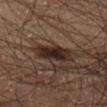workup=catalogued during a skin exam; not biopsied
illumination=cross-polarized illumination
site=the left thigh
image source=total-body-photography crop, ~15 mm field of view
size=~4.5 mm (longest diameter)
subject=male, aged 53 to 57
TBP lesion metrics=a lesion color around L≈23 a*≈14 b*≈19 in CIELAB and a normalized border contrast of about 12.5; a classifier nevus-likeness of about 90/100